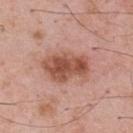No biopsy was performed on this lesion — it was imaged during a full skin examination and was not determined to be concerning.